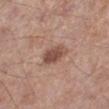• workup · imaged on a skin check; not biopsied
• subject · male, roughly 55 years of age
• body site · the left lower leg
• image · ~15 mm tile from a whole-body skin photo